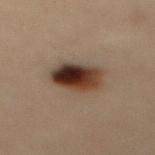| key | value |
|---|---|
| follow-up | catalogued during a skin exam; not biopsied |
| body site | the mid back |
| subject | female, in their 30s |
| tile lighting | cross-polarized illumination |
| lesion diameter | about 4.5 mm |
| image source | 15 mm crop, total-body photography |